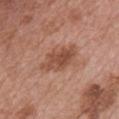Part of a total-body skin-imaging series; this lesion was reviewed on a skin check and was not flagged for biopsy.
Located on the chest.
The patient is a male aged 63–67.
A 15 mm crop from a total-body photograph taken for skin-cancer surveillance.